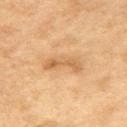notes: catalogued during a skin exam; not biopsied | subject: female, aged approximately 60 | automated metrics: a lesion area of about 7 mm², a shape eccentricity near 0.9, and two-axis asymmetry of about 0.4; a lesion–skin lightness drop of about 8; border irregularity of about 5 on a 0–10 scale and radial color variation of about 1.5; an automated nevus-likeness rating near 5 out of 100 | body site: the left upper arm | lighting: cross-polarized illumination | size: ~4.5 mm (longest diameter) | acquisition: ~15 mm tile from a whole-body skin photo.